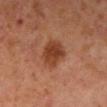Assessment:
Recorded during total-body skin imaging; not selected for excision or biopsy.
Acquisition and patient details:
A lesion tile, about 15 mm wide, cut from a 3D total-body photograph. A male subject about 60 years old. Longest diameter approximately 4 mm. Located on the right lower leg. Captured under cross-polarized illumination. The lesion-visualizer software estimated a border-irregularity index near 1.5/10 and a peripheral color-asymmetry measure near 1.